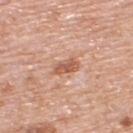  biopsy_status: not biopsied; imaged during a skin examination
  patient:
    sex: male
    age_approx: 80
  lesion_size:
    long_diameter_mm_approx: 3.0
  image:
    source: total-body photography crop
    field_of_view_mm: 15
  automated_metrics:
    cielab_L: 58
    cielab_a: 24
    cielab_b: 32
    vs_skin_darker_L: 12.0
    color_variation_0_10: 2.5
    peripheral_color_asymmetry: 0.5
  lighting: white-light
  site: upper back